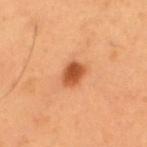No biopsy was performed on this lesion — it was imaged during a full skin examination and was not determined to be concerning.
The lesion is located on the upper back.
Longest diameter approximately 3 mm.
A male subject, approximately 55 years of age.
A 15 mm crop from a total-body photograph taken for skin-cancer surveillance.